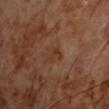{"biopsy_status": "not biopsied; imaged during a skin examination", "site": "chest", "lesion_size": {"long_diameter_mm_approx": 2.5}, "image": {"source": "total-body photography crop", "field_of_view_mm": 15}, "lighting": "cross-polarized", "automated_metrics": {"area_mm2_approx": 2.5, "eccentricity": 0.85, "shape_asymmetry": 0.55, "border_irregularity_0_10": 6.0, "color_variation_0_10": 0.0, "peripheral_color_asymmetry": 0.0}, "patient": {"sex": "male", "age_approx": 60}}